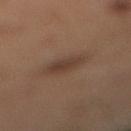The lesion is located on the leg. A male patient, aged 58 to 62. A 15 mm close-up extracted from a 3D total-body photography capture. The lesion-visualizer software estimated a lesion–skin lightness drop of about 7 and a lesion-to-skin contrast of about 6.5 (normalized; higher = more distinct). It also reported an automated nevus-likeness rating near 60 out of 100. About 3 mm across. This is a cross-polarized tile.